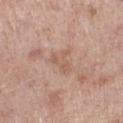Findings:
• lesion size — ~3 mm (longest diameter)
• location — the left lower leg
• automated metrics — an area of roughly 4 mm² and an eccentricity of roughly 0.6; an average lesion color of about L≈58 a*≈19 b*≈29 (CIELAB), about 7 CIELAB-L* units darker than the surrounding skin, and a normalized border contrast of about 5; a border-irregularity index near 7/10 and peripheral color asymmetry of about 0
• illumination — white-light illumination
• subject — female, aged 68–72
• imaging modality — 15 mm crop, total-body photography